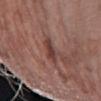workup: total-body-photography surveillance lesion; no biopsy
image source: 15 mm crop, total-body photography
patient: female, aged around 60
location: the arm